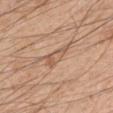<lesion>
<patient>
  <sex>male</sex>
  <age_approx>50</age_approx>
</patient>
<lesion_size>
  <long_diameter_mm_approx>3.5</long_diameter_mm_approx>
</lesion_size>
<site>left upper arm</site>
<automated_metrics>
  <area_mm2_approx>5.0</area_mm2_approx>
  <eccentricity>0.9</eccentricity>
  <shape_asymmetry>0.4</shape_asymmetry>
  <cielab_L>57</cielab_L>
  <cielab_a>19</cielab_a>
  <cielab_b>31</cielab_b>
  <vs_skin_darker_L>8.0</vs_skin_darker_L>
  <vs_skin_contrast_norm>5.5</vs_skin_contrast_norm>
  <border_irregularity_0_10>4.5</border_irregularity_0_10>
  <color_variation_0_10>3.5</color_variation_0_10>
  <peripheral_color_asymmetry>1.0</peripheral_color_asymmetry>
</automated_metrics>
<lighting>white-light</lighting>
<image>
  <source>total-body photography crop</source>
  <field_of_view_mm>15</field_of_view_mm>
</image>
</lesion>